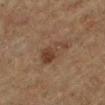biopsy status — catalogued during a skin exam; not biopsied
anatomic site — the left lower leg
subject — male, aged around 85 or older
imaging modality — ~15 mm crop, total-body skin-cancer survey